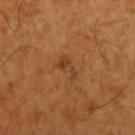This image is a 15 mm lesion crop taken from a total-body photograph.
From the right upper arm.
The patient is a male approximately 65 years of age.